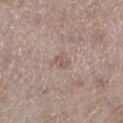The lesion was tiled from a total-body skin photograph and was not biopsied. On the left lower leg. A female patient, about 50 years old. A 15 mm close-up tile from a total-body photography series done for melanoma screening. Automated image analysis of the tile measured a footprint of about 4 mm², an eccentricity of roughly 0.65, and a symmetry-axis asymmetry near 0.3. And it measured a nevus-likeness score of about 0/100 and a detector confidence of about 100 out of 100 that the crop contains a lesion. The tile uses white-light illumination. The recorded lesion diameter is about 2.5 mm.